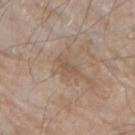Imaged during a routine full-body skin examination; the lesion was not biopsied and no histopathology is available.
Located on the arm.
Automated tile analysis of the lesion measured a border-irregularity rating of about 5.5/10, a within-lesion color-variation index near 1/10, and peripheral color asymmetry of about 0.5.
A male subject, aged approximately 80.
This is a white-light tile.
A region of skin cropped from a whole-body photographic capture, roughly 15 mm wide.
The recorded lesion diameter is about 3.5 mm.